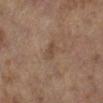| field | value |
|---|---|
| biopsy status | imaged on a skin check; not biopsied |